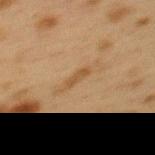| key | value |
|---|---|
| notes | total-body-photography surveillance lesion; no biopsy |
| acquisition | ~15 mm crop, total-body skin-cancer survey |
| tile lighting | cross-polarized |
| location | the upper back |
| automated lesion analysis | a footprint of about 2.5 mm², an outline eccentricity of about 0.95 (0 = round, 1 = elongated), and a shape-asymmetry score of about 0.3 (0 = symmetric); a lesion-to-skin contrast of about 6.5 (normalized; higher = more distinct); a border-irregularity index near 3.5/10 and a color-variation rating of about 0/10 |
| size | about 3 mm |
| subject | female, approximately 40 years of age |